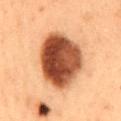Recorded during total-body skin imaging; not selected for excision or biopsy. A male subject aged approximately 55. A region of skin cropped from a whole-body photographic capture, roughly 15 mm wide. The total-body-photography lesion software estimated border irregularity of about 2 on a 0–10 scale, a within-lesion color-variation index near 8.5/10, and a peripheral color-asymmetry measure near 3. The software also gave an automated nevus-likeness rating near 100 out of 100 and lesion-presence confidence of about 100/100. Imaged with cross-polarized lighting. The recorded lesion diameter is about 9 mm. Located on the mid back.